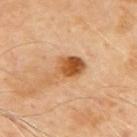location: the upper back
imaging modality: 15 mm crop, total-body photography
lesion size: about 5 mm
automated lesion analysis: a lesion area of about 8 mm², an eccentricity of roughly 0.85, and a symmetry-axis asymmetry near 0.5; border irregularity of about 5.5 on a 0–10 scale, a color-variation rating of about 6/10, and radial color variation of about 2
lighting: cross-polarized illumination
patient: male, roughly 70 years of age